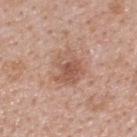Assessment:
Imaged during a routine full-body skin examination; the lesion was not biopsied and no histopathology is available.
Background:
Imaged with white-light lighting. On the upper back. A male patient about 40 years old. Cropped from a total-body skin-imaging series; the visible field is about 15 mm.